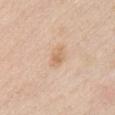  biopsy_status: not biopsied; imaged during a skin examination
  site: chest
  image:
    source: total-body photography crop
    field_of_view_mm: 15
  patient:
    sex: female
    age_approx: 40